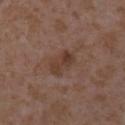| key | value |
|---|---|
| imaging modality | 15 mm crop, total-body photography |
| patient | female, approximately 35 years of age |
| lesion diameter | about 3.5 mm |
| lighting | white-light illumination |
| body site | the right forearm |